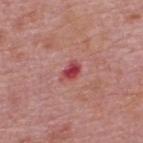Impression:
No biopsy was performed on this lesion — it was imaged during a full skin examination and was not determined to be concerning.
Context:
A 15 mm close-up tile from a total-body photography series done for melanoma screening. A male subject aged 73–77. The lesion is located on the upper back.